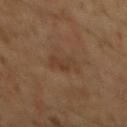The patient is a male aged 58 to 62.
Approximately 3 mm at its widest.
Located on the mid back.
A 15 mm crop from a total-body photograph taken for skin-cancer surveillance.
An algorithmic analysis of the crop reported a footprint of about 5.5 mm², an outline eccentricity of about 0.7 (0 = round, 1 = elongated), and a symmetry-axis asymmetry near 0.25. And it measured a within-lesion color-variation index near 2/10 and peripheral color asymmetry of about 1. It also reported a lesion-detection confidence of about 100/100.